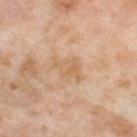Q: Is there a histopathology result?
A: imaged on a skin check; not biopsied
Q: Where on the body is the lesion?
A: the right thigh
Q: Who is the patient?
A: female, aged 53 to 57
Q: Lesion size?
A: about 4 mm
Q: How was this image acquired?
A: ~15 mm tile from a whole-body skin photo
Q: How was the tile lit?
A: cross-polarized illumination